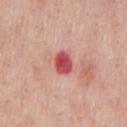<tbp_lesion>
  <biopsy_status>not biopsied; imaged during a skin examination</biopsy_status>
  <lesion_size>
    <long_diameter_mm_approx>2.5</long_diameter_mm_approx>
  </lesion_size>
  <automated_metrics>
    <border_irregularity_0_10>1.5</border_irregularity_0_10>
    <color_variation_0_10>4.0</color_variation_0_10>
    <peripheral_color_asymmetry>1.0</peripheral_color_asymmetry>
    <lesion_detection_confidence_0_100>100</lesion_detection_confidence_0_100>
  </automated_metrics>
  <image>
    <source>total-body photography crop</source>
    <field_of_view_mm>15</field_of_view_mm>
  </image>
  <site>chest</site>
  <patient>
    <sex>male</sex>
    <age_approx>60</age_approx>
  </patient>
</tbp_lesion>